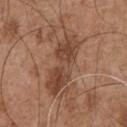notes=no biopsy performed (imaged during a skin exam); imaging modality=15 mm crop, total-body photography; subject=male, in their mid-50s; size=about 11 mm; location=the chest; tile lighting=white-light.